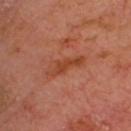Q: Is there a histopathology result?
A: catalogued during a skin exam; not biopsied
Q: What did automated image analysis measure?
A: a mean CIELAB color near L≈44 a*≈28 b*≈35 and roughly 8 lightness units darker than nearby skin
Q: How large is the lesion?
A: about 4.5 mm
Q: Lesion location?
A: the head or neck
Q: What kind of image is this?
A: 15 mm crop, total-body photography
Q: How was the tile lit?
A: cross-polarized
Q: Who is the patient?
A: male, roughly 65 years of age